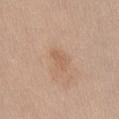| field | value |
|---|---|
| tile lighting | white-light |
| patient | female, aged 18 to 22 |
| image-analysis metrics | an area of roughly 3 mm², an eccentricity of roughly 0.85, and a symmetry-axis asymmetry near 0.4; a mean CIELAB color near L≈59 a*≈19 b*≈32 and roughly 6 lightness units darker than nearby skin; a detector confidence of about 100 out of 100 that the crop contains a lesion |
| image source | 15 mm crop, total-body photography |
| site | the abdomen |
| lesion diameter | about 3 mm |